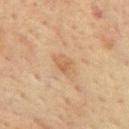Q: Is there a histopathology result?
A: total-body-photography surveillance lesion; no biopsy
Q: Lesion size?
A: about 3 mm
Q: How was this image acquired?
A: ~15 mm crop, total-body skin-cancer survey
Q: Patient demographics?
A: male, aged around 65
Q: Illumination type?
A: cross-polarized illumination
Q: What is the anatomic site?
A: the mid back